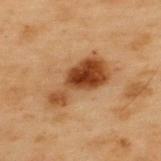Impression:
Imaged during a routine full-body skin examination; the lesion was not biopsied and no histopathology is available.
Background:
The lesion is on the upper back. Captured under cross-polarized illumination. A male subject in their mid-50s. A close-up tile cropped from a whole-body skin photograph, about 15 mm across.